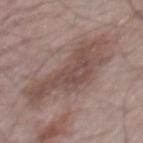Part of a total-body skin-imaging series; this lesion was reviewed on a skin check and was not flagged for biopsy. A 15 mm crop from a total-body photograph taken for skin-cancer surveillance. This is a white-light tile. Approximately 11 mm at its widest. A male subject in their mid-60s. The lesion is on the mid back.